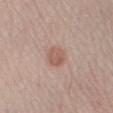notes: imaged on a skin check; not biopsied
diameter: about 2.5 mm
image source: 15 mm crop, total-body photography
patient: female, roughly 45 years of age
site: the left lower leg
image-analysis metrics: a footprint of about 5.5 mm² and two-axis asymmetry of about 0.15; a border-irregularity rating of about 1/10; an automated nevus-likeness rating near 90 out of 100 and a detector confidence of about 100 out of 100 that the crop contains a lesion